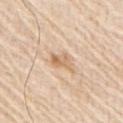Part of a total-body skin-imaging series; this lesion was reviewed on a skin check and was not flagged for biopsy. The patient is a male aged 78–82. Captured under white-light illumination. The lesion is on the right upper arm. The recorded lesion diameter is about 3 mm. Cropped from a total-body skin-imaging series; the visible field is about 15 mm.